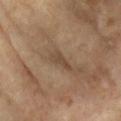biopsy status: catalogued during a skin exam; not biopsied
site: the arm
image source: 15 mm crop, total-body photography
tile lighting: cross-polarized illumination
image-analysis metrics: an area of roughly 3.5 mm², an outline eccentricity of about 0.9 (0 = round, 1 = elongated), and a shape-asymmetry score of about 0.4 (0 = symmetric); a nevus-likeness score of about 0/100 and a lesion-detection confidence of about 80/100
subject: female, aged 73 to 77